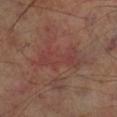| feature | finding |
|---|---|
| follow-up | total-body-photography surveillance lesion; no biopsy |
| diameter | ~6.5 mm (longest diameter) |
| site | the right lower leg |
| image source | ~15 mm tile from a whole-body skin photo |
| tile lighting | cross-polarized illumination |
| patient | male, about 70 years old |